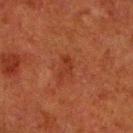Part of a total-body skin-imaging series; this lesion was reviewed on a skin check and was not flagged for biopsy.
The patient is a male in their 80s.
Captured under cross-polarized illumination.
A close-up tile cropped from a whole-body skin photograph, about 15 mm across.
Automated tile analysis of the lesion measured a footprint of about 3 mm², an eccentricity of roughly 0.85, and a symmetry-axis asymmetry near 0.6. The software also gave a nevus-likeness score of about 5/100 and lesion-presence confidence of about 100/100.
On the right lower leg.